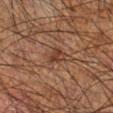biopsy_status: not biopsied; imaged during a skin examination
site: right forearm
patient:
  sex: male
  age_approx: 60
image:
  source: total-body photography crop
  field_of_view_mm: 15